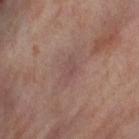Findings:
– patient · female, in their mid- to late 50s
– anatomic site · the right thigh
– acquisition · ~15 mm tile from a whole-body skin photo
– illumination · cross-polarized
– diameter · ~3 mm (longest diameter)